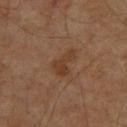Clinical impression: Imaged during a routine full-body skin examination; the lesion was not biopsied and no histopathology is available. Context: Captured under cross-polarized illumination. The total-body-photography lesion software estimated an area of roughly 5.5 mm², an eccentricity of roughly 0.8, and a symmetry-axis asymmetry near 0.55. It also reported a lesion color around L≈34 a*≈18 b*≈28 in CIELAB and roughly 6 lightness units darker than nearby skin. And it measured a border-irregularity rating of about 6/10, internal color variation of about 2 on a 0–10 scale, and a peripheral color-asymmetry measure near 1. This image is a 15 mm lesion crop taken from a total-body photograph. Approximately 3.5 mm at its widest. A male patient aged 58–62. The lesion is located on the chest.